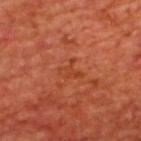Captured during whole-body skin photography for melanoma surveillance; the lesion was not biopsied.